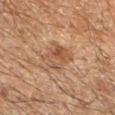The lesion was tiled from a total-body skin photograph and was not biopsied. The lesion is located on the right forearm. A 15 mm crop from a total-body photograph taken for skin-cancer surveillance. This is a cross-polarized tile. A male subject aged around 60.